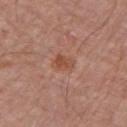lesion diameter: about 2.5 mm | patient: male, about 80 years old | image-analysis metrics: roughly 7 lightness units darker than nearby skin and a lesion-to-skin contrast of about 6.5 (normalized; higher = more distinct); a border-irregularity rating of about 2/10, a color-variation rating of about 2.5/10, and peripheral color asymmetry of about 0.5 | image source: 15 mm crop, total-body photography | lighting: white-light | site: the left upper arm.